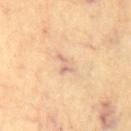Background: The lesion's longest dimension is about 3 mm. A male patient about 70 years old. From the chest. A close-up tile cropped from a whole-body skin photograph, about 15 mm across.